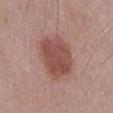Clinical summary:
The lesion's longest dimension is about 5.5 mm. A 15 mm close-up extracted from a 3D total-body photography capture. On the abdomen. Captured under white-light illumination. A male patient, approximately 70 years of age.